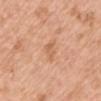Clinical impression:
Imaged during a routine full-body skin examination; the lesion was not biopsied and no histopathology is available.
Context:
A 15 mm close-up extracted from a 3D total-body photography capture. The lesion is on the arm. The total-body-photography lesion software estimated an area of roughly 4 mm², a shape eccentricity near 0.75, and two-axis asymmetry of about 0.35. The tile uses white-light illumination. A female patient roughly 45 years of age. Measured at roughly 2.5 mm in maximum diameter.